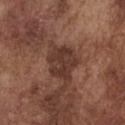biopsy status: total-body-photography surveillance lesion; no biopsy